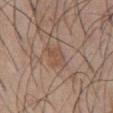Recorded during total-body skin imaging; not selected for excision or biopsy.